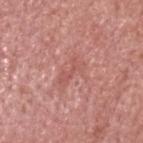Clinical impression:
Captured during whole-body skin photography for melanoma surveillance; the lesion was not biopsied.
Context:
A close-up tile cropped from a whole-body skin photograph, about 15 mm across. Imaged with white-light lighting. Automated tile analysis of the lesion measured a lesion color around L≈55 a*≈27 b*≈26 in CIELAB. It also reported a nevus-likeness score of about 0/100 and a lesion-detection confidence of about 85/100. The recorded lesion diameter is about 3.5 mm. A male subject, aged around 50. Located on the head or neck.